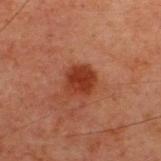{"biopsy_status": "not biopsied; imaged during a skin examination", "site": "back", "image": {"source": "total-body photography crop", "field_of_view_mm": 15}, "patient": {"sex": "male", "age_approx": 60}}